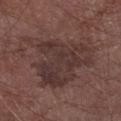Case summary:
• biopsy status · no biopsy performed (imaged during a skin exam)
• subject · male, about 75 years old
• site · the right forearm
• image source · 15 mm crop, total-body photography